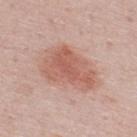Impression:
Recorded during total-body skin imaging; not selected for excision or biopsy.
Clinical summary:
Automated tile analysis of the lesion measured an outline eccentricity of about 0.75 (0 = round, 1 = elongated). And it measured a color-variation rating of about 3.5/10. And it measured a nevus-likeness score of about 95/100 and lesion-presence confidence of about 100/100. The tile uses white-light illumination. A close-up tile cropped from a whole-body skin photograph, about 15 mm across. From the upper back. The patient is a male in their mid-20s. Longest diameter approximately 6 mm.